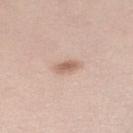Q: Was a biopsy performed?
A: total-body-photography surveillance lesion; no biopsy
Q: How was the tile lit?
A: white-light
Q: Patient demographics?
A: female, aged 38 to 42
Q: Lesion size?
A: ~3 mm (longest diameter)
Q: Lesion location?
A: the right lower leg
Q: What is the imaging modality?
A: 15 mm crop, total-body photography